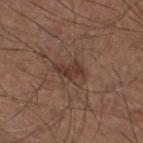Captured during whole-body skin photography for melanoma surveillance; the lesion was not biopsied. The tile uses white-light illumination. A male subject approximately 60 years of age. A lesion tile, about 15 mm wide, cut from a 3D total-body photograph. On the left lower leg. Longest diameter approximately 4 mm.